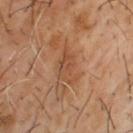The lesion was photographed on a routine skin check and not biopsied; there is no pathology result.